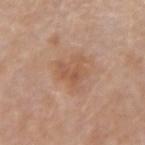| key | value |
|---|---|
| follow-up | no biopsy performed (imaged during a skin exam) |
| lighting | white-light illumination |
| size | ≈4.5 mm |
| body site | the left forearm |
| acquisition | ~15 mm crop, total-body skin-cancer survey |
| patient | female, aged 43 to 47 |